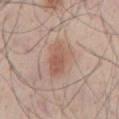biopsy status = imaged on a skin check; not biopsied | imaging modality = 15 mm crop, total-body photography | lesion size = about 5 mm | location = the chest | illumination = white-light | subject = male, aged approximately 30.